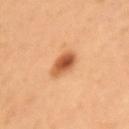Clinical impression: Recorded during total-body skin imaging; not selected for excision or biopsy. Clinical summary: A close-up tile cropped from a whole-body skin photograph, about 15 mm across. On the left upper arm. Captured under cross-polarized illumination. Approximately 3 mm at its widest. The subject is a female in their mid-30s. Automated tile analysis of the lesion measured a footprint of about 7 mm² and an eccentricity of roughly 0.45. And it measured a nevus-likeness score of about 100/100 and lesion-presence confidence of about 100/100.